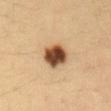Q: Is there a histopathology result?
A: total-body-photography surveillance lesion; no biopsy
Q: Lesion size?
A: about 3.5 mm
Q: How was this image acquired?
A: ~15 mm crop, total-body skin-cancer survey
Q: Where on the body is the lesion?
A: the back
Q: How was the tile lit?
A: cross-polarized illumination
Q: What are the patient's age and sex?
A: male, about 35 years old